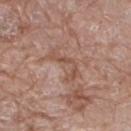This lesion was catalogued during total-body skin photography and was not selected for biopsy. Longest diameter approximately 4.5 mm. Automated tile analysis of the lesion measured a lesion color around L≈51 a*≈20 b*≈27 in CIELAB and a lesion-to-skin contrast of about 6 (normalized; higher = more distinct). It also reported a border-irregularity index near 9.5/10, a color-variation rating of about 0/10, and a peripheral color-asymmetry measure near 0. The patient is a male roughly 80 years of age. The tile uses white-light illumination. A 15 mm close-up tile from a total-body photography series done for melanoma screening. On the right thigh.